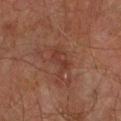Part of a total-body skin-imaging series; this lesion was reviewed on a skin check and was not flagged for biopsy. Cropped from a whole-body photographic skin survey; the tile spans about 15 mm. A male subject, about 75 years old. On the right forearm. Imaged with cross-polarized lighting. The recorded lesion diameter is about 3.5 mm.